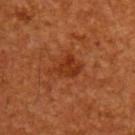workup — imaged on a skin check; not biopsied
automated metrics — a lesion color around L≈32 a*≈28 b*≈35 in CIELAB, a lesion–skin lightness drop of about 7, and a lesion-to-skin contrast of about 7 (normalized; higher = more distinct); a border-irregularity index near 4/10, a within-lesion color-variation index near 2.5/10, and a peripheral color-asymmetry measure near 1
size — about 3.5 mm
location — the upper back
subject — male, roughly 60 years of age
acquisition — total-body-photography crop, ~15 mm field of view